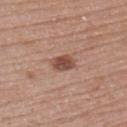Captured during whole-body skin photography for melanoma surveillance; the lesion was not biopsied.
Located on the left upper arm.
Captured under white-light illumination.
The total-body-photography lesion software estimated a footprint of about 4.5 mm² and an eccentricity of roughly 0.75. The analysis additionally found about 12 CIELAB-L* units darker than the surrounding skin and a lesion-to-skin contrast of about 9 (normalized; higher = more distinct). And it measured a border-irregularity index near 2/10, a within-lesion color-variation index near 3/10, and peripheral color asymmetry of about 1.
The patient is a female about 65 years old.
Measured at roughly 3 mm in maximum diameter.
A 15 mm close-up extracted from a 3D total-body photography capture.